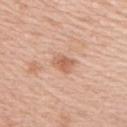No biopsy was performed on this lesion — it was imaged during a full skin examination and was not determined to be concerning.
Located on the upper back.
Cropped from a total-body skin-imaging series; the visible field is about 15 mm.
The subject is a male in their mid- to late 60s.
Measured at roughly 2.5 mm in maximum diameter.